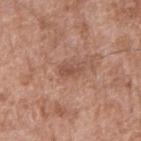notes: catalogued during a skin exam; not biopsied | image: ~15 mm tile from a whole-body skin photo | location: the left upper arm | tile lighting: white-light | subject: male, in their 70s | lesion size: ~2.5 mm (longest diameter) | TBP lesion metrics: an area of roughly 3.5 mm², an outline eccentricity of about 0.85 (0 = round, 1 = elongated), and a symmetry-axis asymmetry near 0.3; a mean CIELAB color near L≈51 a*≈22 b*≈28, roughly 8 lightness units darker than nearby skin, and a lesion-to-skin contrast of about 6 (normalized; higher = more distinct); border irregularity of about 3 on a 0–10 scale and a color-variation rating of about 2/10; a lesion-detection confidence of about 100/100.